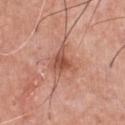Assessment: The lesion was photographed on a routine skin check and not biopsied; there is no pathology result. Acquisition and patient details: The lesion is located on the chest. A 15 mm close-up tile from a total-body photography series done for melanoma screening. This is a white-light tile. The subject is a male aged around 60.